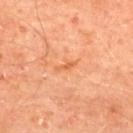{"biopsy_status": "not biopsied; imaged during a skin examination", "patient": {"sex": "male", "age_approx": 65}, "lesion_size": {"long_diameter_mm_approx": 2.5}, "automated_metrics": {"cielab_L": 63, "cielab_a": 30, "cielab_b": 43, "vs_skin_contrast_norm": 6.0, "border_irregularity_0_10": 5.0, "color_variation_0_10": 0.0, "lesion_detection_confidence_0_100": 100}, "image": {"source": "total-body photography crop", "field_of_view_mm": 15}, "site": "upper back"}